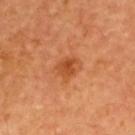{
  "biopsy_status": "not biopsied; imaged during a skin examination",
  "lesion_size": {
    "long_diameter_mm_approx": 3.0
  },
  "image": {
    "source": "total-body photography crop",
    "field_of_view_mm": 15
  },
  "site": "upper back",
  "patient": {
    "sex": "male",
    "age_approx": 65
  },
  "automated_metrics": {
    "area_mm2_approx": 6.0,
    "shape_asymmetry": 0.25,
    "cielab_L": 51,
    "cielab_a": 30,
    "cielab_b": 42,
    "vs_skin_darker_L": 9.0,
    "vs_skin_contrast_norm": 7.0,
    "border_irregularity_0_10": 2.5,
    "color_variation_0_10": 4.0,
    "peripheral_color_asymmetry": 1.5
  },
  "lighting": "cross-polarized"
}